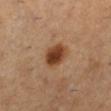Impression:
The lesion was photographed on a routine skin check and not biopsied; there is no pathology result.
Acquisition and patient details:
A female subject in their 30s. A 15 mm close-up tile from a total-body photography series done for melanoma screening. The lesion is on the leg. Captured under cross-polarized illumination. Measured at roughly 3.5 mm in maximum diameter.